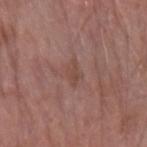biopsy status: no biopsy performed (imaged during a skin exam) | diameter: ~2.5 mm (longest diameter) | site: the left forearm | TBP lesion metrics: an area of roughly 2.5 mm², a shape eccentricity near 0.85, and a shape-asymmetry score of about 0.3 (0 = symmetric); an average lesion color of about L≈45 a*≈21 b*≈23 (CIELAB) and a lesion-to-skin contrast of about 5 (normalized; higher = more distinct); a lesion-detection confidence of about 100/100 | patient: male, aged 78 to 82 | image: ~15 mm tile from a whole-body skin photo.